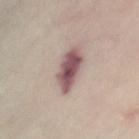• notes: total-body-photography surveillance lesion; no biopsy
• site: the chest
• image: ~15 mm tile from a whole-body skin photo
• patient: female, aged 53 to 57